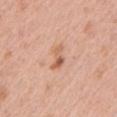Part of a total-body skin-imaging series; this lesion was reviewed on a skin check and was not flagged for biopsy. The recorded lesion diameter is about 3.5 mm. A 15 mm close-up extracted from a 3D total-body photography capture. The lesion is on the left upper arm. The total-body-photography lesion software estimated a lesion color around L≈61 a*≈23 b*≈34 in CIELAB, a lesion–skin lightness drop of about 11, and a normalized lesion–skin contrast near 7. The analysis additionally found border irregularity of about 5 on a 0–10 scale. The analysis additionally found a classifier nevus-likeness of about 25/100 and a detector confidence of about 100 out of 100 that the crop contains a lesion. A female patient approximately 45 years of age.